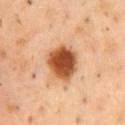Notes:
• workup · imaged on a skin check; not biopsied
• body site · the front of the torso
• acquisition · ~15 mm crop, total-body skin-cancer survey
• patient · male, aged 48 to 52